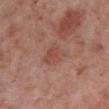follow-up: catalogued during a skin exam; not biopsied | site: the leg | image source: ~15 mm tile from a whole-body skin photo | tile lighting: white-light | subject: male, approximately 70 years of age | size: ≈4 mm | TBP lesion metrics: an area of roughly 9 mm², a shape eccentricity near 0.65, and a shape-asymmetry score of about 0.25 (0 = symmetric); an average lesion color of about L≈49 a*≈22 b*≈26 (CIELAB), roughly 7 lightness units darker than nearby skin, and a lesion-to-skin contrast of about 5.5 (normalized; higher = more distinct); border irregularity of about 2.5 on a 0–10 scale.